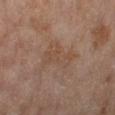Clinical impression: Part of a total-body skin-imaging series; this lesion was reviewed on a skin check and was not flagged for biopsy.